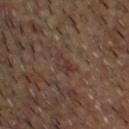Recorded during total-body skin imaging; not selected for excision or biopsy. Cropped from a total-body skin-imaging series; the visible field is about 15 mm. The lesion's longest dimension is about 3 mm. Automated tile analysis of the lesion measured a lesion area of about 3.5 mm², a shape eccentricity near 0.85, and a shape-asymmetry score of about 0.35 (0 = symmetric). And it measured border irregularity of about 3.5 on a 0–10 scale, a within-lesion color-variation index near 3.5/10, and peripheral color asymmetry of about 1. A male subject, roughly 60 years of age. The lesion is on the head or neck.